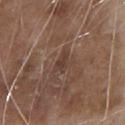Q: Illumination type?
A: white-light
Q: What kind of image is this?
A: ~15 mm tile from a whole-body skin photo
Q: What are the patient's age and sex?
A: male, aged 78–82
Q: How large is the lesion?
A: about 2.5 mm
Q: Where on the body is the lesion?
A: the chest
Q: What did automated image analysis measure?
A: an area of roughly 3.5 mm² and two-axis asymmetry of about 0.35; an average lesion color of about L≈39 a*≈17 b*≈23 (CIELAB) and roughly 7 lightness units darker than nearby skin